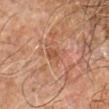The tile uses cross-polarized illumination.
An algorithmic analysis of the crop reported a lesion color around L≈42 a*≈19 b*≈28 in CIELAB, about 6 CIELAB-L* units darker than the surrounding skin, and a lesion-to-skin contrast of about 5 (normalized; higher = more distinct).
A male patient, in their 70s.
On the left forearm.
The recorded lesion diameter is about 3 mm.
A region of skin cropped from a whole-body photographic capture, roughly 15 mm wide.